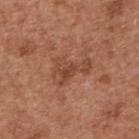Assessment:
Part of a total-body skin-imaging series; this lesion was reviewed on a skin check and was not flagged for biopsy.
Clinical summary:
This is a white-light tile. The lesion is on the upper back. A lesion tile, about 15 mm wide, cut from a 3D total-body photograph. Automated tile analysis of the lesion measured a border-irregularity index near 6.5/10, a color-variation rating of about 3.5/10, and a peripheral color-asymmetry measure near 1. A male patient, in their mid-60s.